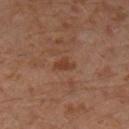Clinical impression: Recorded during total-body skin imaging; not selected for excision or biopsy. Background: The lesion-visualizer software estimated a color-variation rating of about 0.5/10 and peripheral color asymmetry of about 0. It also reported an automated nevus-likeness rating near 0 out of 100 and a lesion-detection confidence of about 100/100. A 15 mm close-up tile from a total-body photography series done for melanoma screening. A male subject, approximately 30 years of age. The lesion is on the left lower leg.